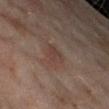Assessment:
Captured during whole-body skin photography for melanoma surveillance; the lesion was not biopsied.
Context:
This is a cross-polarized tile. The lesion is located on the right lower leg. A female patient about 60 years old. A lesion tile, about 15 mm wide, cut from a 3D total-body photograph. Automated tile analysis of the lesion measured a lesion area of about 4.5 mm², a shape eccentricity near 0.7, and a shape-asymmetry score of about 0.3 (0 = symmetric). It also reported an average lesion color of about L≈35 a*≈15 b*≈21 (CIELAB), roughly 5 lightness units darker than nearby skin, and a normalized border contrast of about 5. About 2.5 mm across.